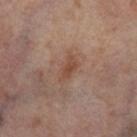Part of a total-body skin-imaging series; this lesion was reviewed on a skin check and was not flagged for biopsy.
The lesion is on the right thigh.
A region of skin cropped from a whole-body photographic capture, roughly 15 mm wide.
About 3.5 mm across.
The subject is a female roughly 55 years of age.
This is a cross-polarized tile.